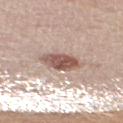This lesion was catalogued during total-body skin photography and was not selected for biopsy.
A 15 mm crop from a total-body photograph taken for skin-cancer surveillance.
This is a white-light tile.
A male patient, aged 73–77.
On the right upper arm.